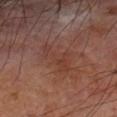workup — total-body-photography surveillance lesion; no biopsy | body site — the left forearm | lesion size — about 5 mm | subject — male, aged 68–72 | automated metrics — an area of roughly 7 mm², an outline eccentricity of about 0.9 (0 = round, 1 = elongated), and a symmetry-axis asymmetry near 0.55; a mean CIELAB color near L≈37 a*≈22 b*≈25 and roughly 5 lightness units darker than nearby skin; a classifier nevus-likeness of about 0/100 and lesion-presence confidence of about 100/100 | acquisition — ~15 mm crop, total-body skin-cancer survey | illumination — cross-polarized.